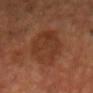subject: male, aged 53–57 | image-analysis metrics: a mean CIELAB color near L≈34 a*≈22 b*≈30, roughly 6 lightness units darker than nearby skin, and a lesion-to-skin contrast of about 6 (normalized; higher = more distinct); a border-irregularity rating of about 2.5/10, a color-variation rating of about 3.5/10, and radial color variation of about 1 | lesion size: ~7 mm (longest diameter) | imaging modality: ~15 mm tile from a whole-body skin photo | tile lighting: cross-polarized | location: the head or neck.